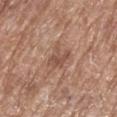image source: total-body-photography crop, ~15 mm field of view; site: the left lower leg; patient: female, about 75 years old.